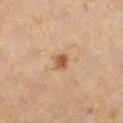Clinical impression: Imaged during a routine full-body skin examination; the lesion was not biopsied and no histopathology is available. Clinical summary: The tile uses cross-polarized illumination. The lesion is located on the right lower leg. Approximately 2 mm at its widest. A female patient, aged 43–47. A close-up tile cropped from a whole-body skin photograph, about 15 mm across.